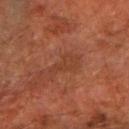Recorded during total-body skin imaging; not selected for excision or biopsy.